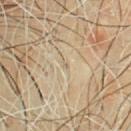workup = imaged on a skin check; not biopsied
automated metrics = a lesion–skin lightness drop of about 5; border irregularity of about 3 on a 0–10 scale, internal color variation of about 0 on a 0–10 scale, and a peripheral color-asymmetry measure near 0; a nevus-likeness score of about 0/100
lesion diameter = about 1 mm
image source = ~15 mm tile from a whole-body skin photo
illumination = cross-polarized illumination
location = the chest
patient = male, aged approximately 45